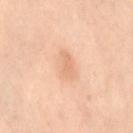Q: Was this lesion biopsied?
A: catalogued during a skin exam; not biopsied
Q: What are the patient's age and sex?
A: female, aged 38–42
Q: What is the imaging modality?
A: ~15 mm crop, total-body skin-cancer survey
Q: What is the anatomic site?
A: the lower back
Q: What is the lesion's diameter?
A: ~3.5 mm (longest diameter)